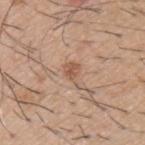Imaged during a routine full-body skin examination; the lesion was not biopsied and no histopathology is available. Imaged with white-light lighting. The recorded lesion diameter is about 2.5 mm. The lesion is on the upper back. Automated tile analysis of the lesion measured a lesion–skin lightness drop of about 9 and a lesion-to-skin contrast of about 6.5 (normalized; higher = more distinct). The software also gave a nevus-likeness score of about 25/100 and a detector confidence of about 100 out of 100 that the crop contains a lesion. A close-up tile cropped from a whole-body skin photograph, about 15 mm across. A male subject, approximately 60 years of age.